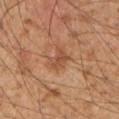follow-up: imaged on a skin check; not biopsied
diameter: about 3.5 mm
image-analysis metrics: a color-variation rating of about 2/10 and radial color variation of about 0.5; lesion-presence confidence of about 100/100
acquisition: 15 mm crop, total-body photography
patient: male, aged around 50
site: the arm
illumination: cross-polarized